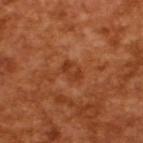Recorded during total-body skin imaging; not selected for excision or biopsy.